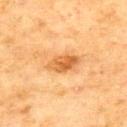• biopsy status: imaged on a skin check; not biopsied
• TBP lesion metrics: a lesion area of about 7 mm², an outline eccentricity of about 0.85 (0 = round, 1 = elongated), and a shape-asymmetry score of about 0.2 (0 = symmetric); roughly 11 lightness units darker than nearby skin and a normalized border contrast of about 8; a border-irregularity rating of about 2.5/10, a color-variation rating of about 4.5/10, and a peripheral color-asymmetry measure near 1.5; a classifier nevus-likeness of about 75/100 and lesion-presence confidence of about 100/100
• image source: ~15 mm tile from a whole-body skin photo
• lighting: cross-polarized illumination
• lesion diameter: ≈4 mm
• subject: male, roughly 70 years of age
• location: the upper back